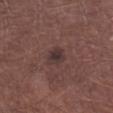  biopsy_status: not biopsied; imaged during a skin examination
  image:
    source: total-body photography crop
    field_of_view_mm: 15
  site: right lower leg
  lesion_size:
    long_diameter_mm_approx: 3.0
  patient:
    sex: male
    age_approx: 55